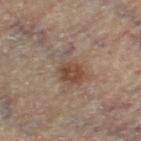lesion_size:
  long_diameter_mm_approx: 5.0
patient:
  sex: male
  age_approx: 85+
automated_metrics:
  border_irregularity_0_10: 5.5
  color_variation_0_10: 6.5
  peripheral_color_asymmetry: 2.0
  lesion_detection_confidence_0_100: 100
site: left thigh
image:
  source: total-body photography crop
  field_of_view_mm: 15
lighting: cross-polarized